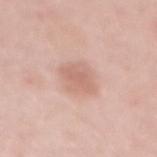Findings:
• notes — total-body-photography surveillance lesion; no biopsy
• lighting — white-light illumination
• subject — female, aged approximately 50
• location — the back
• image source — ~15 mm tile from a whole-body skin photo